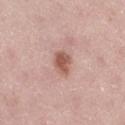biopsy status: imaged on a skin check; not biopsied | subject: female, roughly 50 years of age | site: the right thigh | imaging modality: ~15 mm crop, total-body skin-cancer survey.